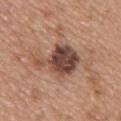Q: Was this lesion biopsied?
A: imaged on a skin check; not biopsied
Q: What did automated image analysis measure?
A: a lesion area of about 15 mm², an outline eccentricity of about 0.75 (0 = round, 1 = elongated), and two-axis asymmetry of about 0.35; an average lesion color of about L≈45 a*≈20 b*≈25 (CIELAB), about 15 CIELAB-L* units darker than the surrounding skin, and a normalized lesion–skin contrast near 11; a border-irregularity index near 5/10
Q: Lesion location?
A: the back
Q: Illumination type?
A: white-light
Q: Patient demographics?
A: male, approximately 50 years of age
Q: What is the imaging modality?
A: ~15 mm crop, total-body skin-cancer survey